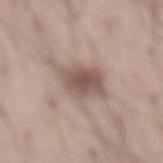<case>
<biopsy_status>not biopsied; imaged during a skin examination</biopsy_status>
<lighting>white-light</lighting>
<lesion_size>
  <long_diameter_mm_approx>6.0</long_diameter_mm_approx>
</lesion_size>
<automated_metrics>
  <area_mm2_approx>13.0</area_mm2_approx>
  <eccentricity>0.75</eccentricity>
  <shape_asymmetry>0.25</shape_asymmetry>
  <border_irregularity_0_10>4.0</border_irregularity_0_10>
  <color_variation_0_10>4.0</color_variation_0_10>
  <peripheral_color_asymmetry>1.0</peripheral_color_asymmetry>
  <nevus_likeness_0_100>85</nevus_likeness_0_100>
  <lesion_detection_confidence_0_100>100</lesion_detection_confidence_0_100>
</automated_metrics>
<patient>
  <sex>male</sex>
  <age_approx>55</age_approx>
</patient>
<site>abdomen</site>
<image>
  <source>total-body photography crop</source>
  <field_of_view_mm>15</field_of_view_mm>
</image>
</case>